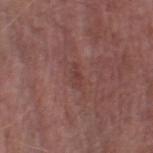<record>
<biopsy_status>not biopsied; imaged during a skin examination</biopsy_status>
<site>right thigh</site>
<automated_metrics>
  <cielab_L>40</cielab_L>
  <cielab_a>22</cielab_a>
  <cielab_b>22</cielab_b>
  <vs_skin_darker_L>6.0</vs_skin_darker_L>
</automated_metrics>
<patient>
  <sex>male</sex>
  <age_approx>75</age_approx>
</patient>
<image>
  <source>total-body photography crop</source>
  <field_of_view_mm>15</field_of_view_mm>
</image>
<lesion_size>
  <long_diameter_mm_approx>2.5</long_diameter_mm_approx>
</lesion_size>
<lighting>white-light</lighting>
</record>